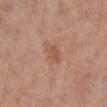{"site": "leg", "automated_metrics": {"area_mm2_approx": 4.0, "eccentricity": 0.8, "shape_asymmetry": 0.2, "cielab_L": 54, "cielab_a": 22, "cielab_b": 31, "vs_skin_contrast_norm": 5.5}, "image": {"source": "total-body photography crop", "field_of_view_mm": 15}, "lighting": "white-light", "patient": {"sex": "female", "age_approx": 40}}